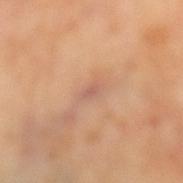Clinical impression: Recorded during total-body skin imaging; not selected for excision or biopsy. Acquisition and patient details: This image is a 15 mm lesion crop taken from a total-body photograph. The patient is a female aged 48 to 52. The lesion is on the left lower leg.